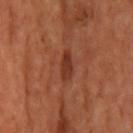No biopsy was performed on this lesion — it was imaged during a full skin examination and was not determined to be concerning. The lesion's longest dimension is about 3.5 mm. Automated tile analysis of the lesion measured a border-irregularity index near 2.5/10 and a within-lesion color-variation index near 1.5/10. The analysis additionally found a classifier nevus-likeness of about 55/100. The tile uses cross-polarized illumination. On the head or neck. Cropped from a whole-body photographic skin survey; the tile spans about 15 mm. A male subject, roughly 65 years of age.